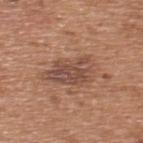Located on the upper back.
Automated tile analysis of the lesion measured a footprint of about 14 mm², an outline eccentricity of about 0.8 (0 = round, 1 = elongated), and a shape-asymmetry score of about 0.3 (0 = symmetric). The analysis additionally found border irregularity of about 3.5 on a 0–10 scale, a color-variation rating of about 5/10, and peripheral color asymmetry of about 1.5.
This is a white-light tile.
A 15 mm close-up tile from a total-body photography series done for melanoma screening.
A male patient aged around 65.